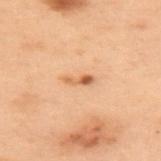A female subject, aged around 55.
Located on the back.
Automated tile analysis of the lesion measured an area of roughly 3 mm², an eccentricity of roughly 0.95, and two-axis asymmetry of about 0.25. And it measured roughly 10 lightness units darker than nearby skin and a lesion-to-skin contrast of about 7 (normalized; higher = more distinct). It also reported a color-variation rating of about 1.5/10 and peripheral color asymmetry of about 0.5. The analysis additionally found a nevus-likeness score of about 85/100 and lesion-presence confidence of about 100/100.
A lesion tile, about 15 mm wide, cut from a 3D total-body photograph.
The recorded lesion diameter is about 3 mm.
Imaged with cross-polarized lighting.